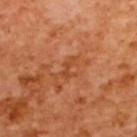Assessment: Recorded during total-body skin imaging; not selected for excision or biopsy. Image and clinical context: A 15 mm crop from a total-body photograph taken for skin-cancer surveillance. A female patient, aged 53–57. This is a cross-polarized tile. The lesion is on the upper back.